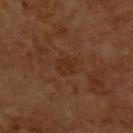biopsy_status: not biopsied; imaged during a skin examination
site: upper back
patient:
  sex: male
  age_approx: 60
lesion_size:
  long_diameter_mm_approx: 2.5
image:
  source: total-body photography crop
  field_of_view_mm: 15